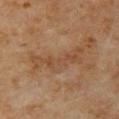Clinical impression:
Part of a total-body skin-imaging series; this lesion was reviewed on a skin check and was not flagged for biopsy.
Acquisition and patient details:
From the upper back. The patient is a male aged 58–62. A 15 mm close-up tile from a total-body photography series done for melanoma screening.